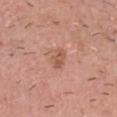Imaged during a routine full-body skin examination; the lesion was not biopsied and no histopathology is available.
The patient is a male aged 53 to 57.
From the head or neck.
A roughly 15 mm field-of-view crop from a total-body skin photograph.
The lesion-visualizer software estimated an area of roughly 4 mm². The software also gave internal color variation of about 3.5 on a 0–10 scale and a peripheral color-asymmetry measure near 1.5.